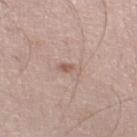<tbp_lesion>
  <biopsy_status>not biopsied; imaged during a skin examination</biopsy_status>
</tbp_lesion>